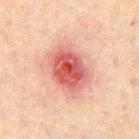notes: imaged on a skin check; not biopsied
subject: male, aged approximately 60
body site: the chest
image source: ~15 mm tile from a whole-body skin photo